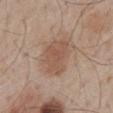Assessment:
Recorded during total-body skin imaging; not selected for excision or biopsy.
Context:
Imaged with white-light lighting. A male subject in their mid-50s. The lesion is on the back. Longest diameter approximately 4.5 mm. A roughly 15 mm field-of-view crop from a total-body skin photograph.